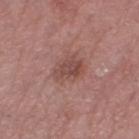The lesion was photographed on a routine skin check and not biopsied; there is no pathology result. Automated image analysis of the tile measured an outline eccentricity of about 0.7 (0 = round, 1 = elongated) and a symmetry-axis asymmetry near 0.4. And it measured an average lesion color of about L≈47 a*≈22 b*≈23 (CIELAB) and about 9 CIELAB-L* units darker than the surrounding skin. It also reported a border-irregularity rating of about 4.5/10 and peripheral color asymmetry of about 1. And it measured an automated nevus-likeness rating near 10 out of 100 and a lesion-detection confidence of about 100/100. Captured under white-light illumination. The lesion is located on the right thigh. Longest diameter approximately 3.5 mm. Cropped from a total-body skin-imaging series; the visible field is about 15 mm. A female subject, roughly 70 years of age.